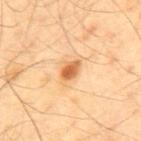No biopsy was performed on this lesion — it was imaged during a full skin examination and was not determined to be concerning. Located on the mid back. Cropped from a whole-body photographic skin survey; the tile spans about 15 mm. Automated tile analysis of the lesion measured an area of roughly 4 mm², an outline eccentricity of about 0.75 (0 = round, 1 = elongated), and two-axis asymmetry of about 0.3. Imaged with cross-polarized lighting. A male patient approximately 40 years of age.